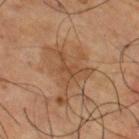Case summary:
• biopsy status: total-body-photography surveillance lesion; no biopsy
• size: ≈5 mm
• imaging modality: 15 mm crop, total-body photography
• location: the right thigh
• patient: male, aged around 65